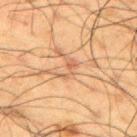The lesion was photographed on a routine skin check and not biopsied; there is no pathology result. On the back. Captured under cross-polarized illumination. A 15 mm close-up extracted from a 3D total-body photography capture. The subject is a male approximately 60 years of age. Measured at roughly 2.5 mm in maximum diameter. An algorithmic analysis of the crop reported an eccentricity of roughly 0.8 and a symmetry-axis asymmetry near 0.55. The analysis additionally found a border-irregularity index near 7.5/10, a color-variation rating of about 0.5/10, and a peripheral color-asymmetry measure near 0.